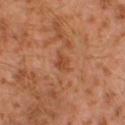No biopsy was performed on this lesion — it was imaged during a full skin examination and was not determined to be concerning. The lesion is located on the left lower leg. A region of skin cropped from a whole-body photographic capture, roughly 15 mm wide. The tile uses cross-polarized illumination. The subject is a male approximately 30 years of age. Automated image analysis of the tile measured border irregularity of about 3.5 on a 0–10 scale, a within-lesion color-variation index near 2/10, and radial color variation of about 0.5. The analysis additionally found a nevus-likeness score of about 0/100. Approximately 2.5 mm at its widest.